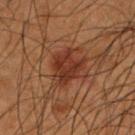Captured during whole-body skin photography for melanoma surveillance; the lesion was not biopsied.
A male patient, aged 48 to 52.
The lesion's longest dimension is about 4.5 mm.
A lesion tile, about 15 mm wide, cut from a 3D total-body photograph.
From the left upper arm.
An algorithmic analysis of the crop reported a footprint of about 11 mm² and a shape eccentricity near 0.55. The analysis additionally found a lesion color around L≈26 a*≈20 b*≈24 in CIELAB and about 8 CIELAB-L* units darker than the surrounding skin. It also reported an automated nevus-likeness rating near 35 out of 100.
Captured under cross-polarized illumination.